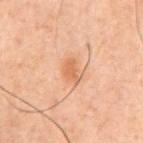Imaged during a routine full-body skin examination; the lesion was not biopsied and no histopathology is available.
Imaged with cross-polarized lighting.
Automated tile analysis of the lesion measured a mean CIELAB color near L≈49 a*≈20 b*≈31, about 7 CIELAB-L* units darker than the surrounding skin, and a normalized border contrast of about 6.5. The software also gave a within-lesion color-variation index near 1/10 and a peripheral color-asymmetry measure near 0.5.
Approximately 3 mm at its widest.
A roughly 15 mm field-of-view crop from a total-body skin photograph.
The lesion is on the front of the torso.
A male patient, aged around 60.